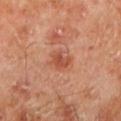Q: Was this lesion biopsied?
A: no biopsy performed (imaged during a skin exam)
Q: How large is the lesion?
A: about 2.5 mm
Q: Illumination type?
A: cross-polarized illumination
Q: Who is the patient?
A: male, aged 68–72
Q: What kind of image is this?
A: total-body-photography crop, ~15 mm field of view
Q: Lesion location?
A: the left lower leg